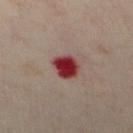Clinical impression: Recorded during total-body skin imaging; not selected for excision or biopsy. Clinical summary: Located on the chest. A female subject roughly 45 years of age. Longest diameter approximately 3.5 mm. A lesion tile, about 15 mm wide, cut from a 3D total-body photograph. The tile uses cross-polarized illumination.